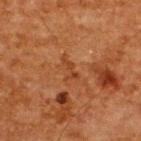Q: Was this lesion biopsied?
A: no biopsy performed (imaged during a skin exam)